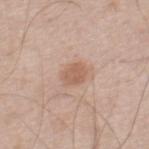* workup · imaged on a skin check; not biopsied
* site · the leg
* image source · ~15 mm crop, total-body skin-cancer survey
* size · about 2.5 mm
* illumination · white-light illumination
* automated lesion analysis · border irregularity of about 2.5 on a 0–10 scale and a within-lesion color-variation index near 1.5/10
* patient · male, in their 60s